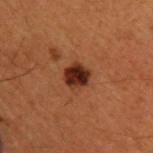The lesion was tiled from a total-body skin photograph and was not biopsied. From the back. The lesion's longest dimension is about 3 mm. This is a cross-polarized tile. A male patient about 50 years old. A 15 mm close-up tile from a total-body photography series done for melanoma screening.